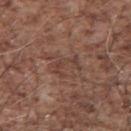The tile uses white-light illumination. A 15 mm close-up tile from a total-body photography series done for melanoma screening. Located on the mid back. The lesion's longest dimension is about 3 mm. A male subject in their mid- to late 50s.